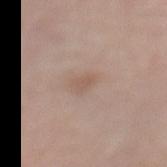Clinical impression:
Recorded during total-body skin imaging; not selected for excision or biopsy.
Image and clinical context:
Automated image analysis of the tile measured an eccentricity of roughly 0.8 and a symmetry-axis asymmetry near 0.3. It also reported an average lesion color of about L≈56 a*≈17 b*≈26 (CIELAB), a lesion–skin lightness drop of about 7, and a lesion-to-skin contrast of about 5 (normalized; higher = more distinct). The analysis additionally found a border-irregularity index near 3/10 and a within-lesion color-variation index near 1/10. This is a white-light tile. Measured at roughly 2.5 mm in maximum diameter. From the left lower leg. A roughly 15 mm field-of-view crop from a total-body skin photograph. A male patient aged 68 to 72.